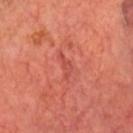follow-up: catalogued during a skin exam; not biopsied | patient: male, aged 63 to 67 | lesion diameter: ≈3 mm | image source: ~15 mm crop, total-body skin-cancer survey.